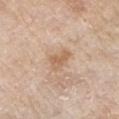{
  "biopsy_status": "not biopsied; imaged during a skin examination",
  "site": "arm",
  "image": {
    "source": "total-body photography crop",
    "field_of_view_mm": 15
  },
  "lighting": "white-light",
  "patient": {
    "sex": "male",
    "age_approx": 65
  },
  "lesion_size": {
    "long_diameter_mm_approx": 3.0
  }
}